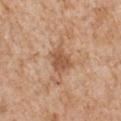notes: no biopsy performed (imaged during a skin exam) | lesion diameter: ≈3 mm | tile lighting: white-light illumination | subject: male, aged around 65 | body site: the left upper arm | imaging modality: ~15 mm crop, total-body skin-cancer survey | automated metrics: a footprint of about 6.5 mm², an outline eccentricity of about 0.65 (0 = round, 1 = elongated), and a shape-asymmetry score of about 0.2 (0 = symmetric); a border-irregularity index near 2/10 and peripheral color asymmetry of about 1; lesion-presence confidence of about 100/100.